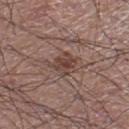Imaged during a routine full-body skin examination; the lesion was not biopsied and no histopathology is available. A male patient, aged around 60. A roughly 15 mm field-of-view crop from a total-body skin photograph. The lesion is on the right lower leg.